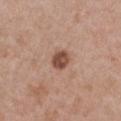Impression: The lesion was photographed on a routine skin check and not biopsied; there is no pathology result. Acquisition and patient details: From the chest. A lesion tile, about 15 mm wide, cut from a 3D total-body photograph. A female patient approximately 30 years of age.